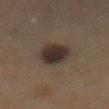Clinical impression:
Part of a total-body skin-imaging series; this lesion was reviewed on a skin check and was not flagged for biopsy.
Acquisition and patient details:
Cropped from a whole-body photographic skin survey; the tile spans about 15 mm. A female subject, in their 60s. Located on the abdomen. The recorded lesion diameter is about 5 mm.